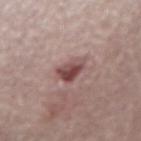– workup: catalogued during a skin exam; not biopsied
– site: the arm
– image source: ~15 mm crop, total-body skin-cancer survey
– automated lesion analysis: an outline eccentricity of about 0.65 (0 = round, 1 = elongated) and two-axis asymmetry of about 0.3; a lesion color around L≈46 a*≈21 b*≈20 in CIELAB, roughly 13 lightness units darker than nearby skin, and a normalized border contrast of about 9.5
– lesion diameter: about 3.5 mm
– subject: male, approximately 65 years of age
– lighting: white-light